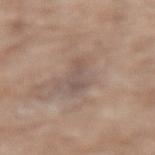workup — catalogued during a skin exam; not biopsied | lighting — white-light | acquisition — 15 mm crop, total-body photography | patient — male, aged approximately 80 | site — the lower back | diameter — ≈4 mm.